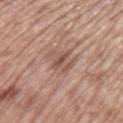Imaged during a routine full-body skin examination; the lesion was not biopsied and no histopathology is available.
Located on the left upper arm.
A male patient, aged 68 to 72.
Captured under white-light illumination.
A 15 mm close-up tile from a total-body photography series done for melanoma screening.